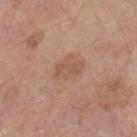{"biopsy_status": "not biopsied; imaged during a skin examination", "image": {"source": "total-body photography crop", "field_of_view_mm": 15}, "patient": {"sex": "male", "age_approx": 70}, "lesion_size": {"long_diameter_mm_approx": 3.5}, "lighting": "white-light", "automated_metrics": {"cielab_L": 55, "cielab_a": 20, "cielab_b": 28, "vs_skin_darker_L": 7.0, "nevus_likeness_0_100": 5, "lesion_detection_confidence_0_100": 100}, "site": "leg"}